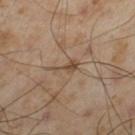Impression:
Imaged during a routine full-body skin examination; the lesion was not biopsied and no histopathology is available.
Acquisition and patient details:
A roughly 15 mm field-of-view crop from a total-body skin photograph. From the left thigh. The total-body-photography lesion software estimated an area of roughly 3.5 mm², an outline eccentricity of about 0.85 (0 = round, 1 = elongated), and a symmetry-axis asymmetry near 0.55. The analysis additionally found roughly 8 lightness units darker than nearby skin and a normalized border contrast of about 6.5. And it measured a border-irregularity index near 6/10, a within-lesion color-variation index near 2/10, and peripheral color asymmetry of about 0.5. Captured under cross-polarized illumination. The lesion's longest dimension is about 3 mm. A male patient, in their mid- to late 50s.